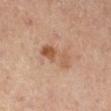Assessment:
The lesion was photographed on a routine skin check and not biopsied; there is no pathology result.